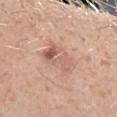Recorded during total-body skin imaging; not selected for excision or biopsy. A male subject aged 28 to 32. An algorithmic analysis of the crop reported a lesion area of about 10 mm² and a symmetry-axis asymmetry near 0.25. The analysis additionally found a lesion color around L≈61 a*≈22 b*≈27 in CIELAB, roughly 9 lightness units darker than nearby skin, and a normalized lesion–skin contrast near 6. The software also gave a border-irregularity rating of about 3/10, a color-variation rating of about 8.5/10, and peripheral color asymmetry of about 3. Longest diameter approximately 4.5 mm. From the left forearm. This image is a 15 mm lesion crop taken from a total-body photograph.